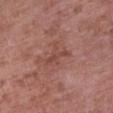Captured during whole-body skin photography for melanoma surveillance; the lesion was not biopsied. Approximately 3.5 mm at its widest. Captured under white-light illumination. An algorithmic analysis of the crop reported a mean CIELAB color near L≈46 a*≈24 b*≈25, a lesion–skin lightness drop of about 7, and a lesion-to-skin contrast of about 5.5 (normalized; higher = more distinct). The software also gave a classifier nevus-likeness of about 0/100 and lesion-presence confidence of about 95/100. The lesion is located on the right upper arm. A roughly 15 mm field-of-view crop from a total-body skin photograph. A female patient aged 68–72.